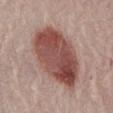acquisition — ~15 mm tile from a whole-body skin photo | location — the abdomen | subject — female, aged around 65 | illumination — white-light | diameter — ~8.5 mm (longest diameter).